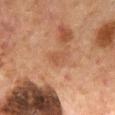- notes · no biopsy performed (imaged during a skin exam)
- lighting · cross-polarized illumination
- anatomic site · the chest
- patient · male, about 65 years old
- lesion size · ≈2.5 mm
- acquisition · total-body-photography crop, ~15 mm field of view